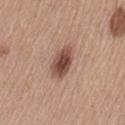Imaged during a routine full-body skin examination; the lesion was not biopsied and no histopathology is available. A close-up tile cropped from a whole-body skin photograph, about 15 mm across. About 4 mm across. Imaged with white-light lighting. Located on the lower back. The subject is a female about 55 years old.